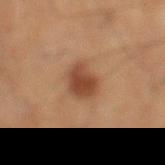Captured during whole-body skin photography for melanoma surveillance; the lesion was not biopsied. This is a cross-polarized tile. A male patient aged around 60. Automated tile analysis of the lesion measured a lesion area of about 9 mm², an outline eccentricity of about 0.7 (0 = round, 1 = elongated), and a symmetry-axis asymmetry near 0.2. The software also gave a lesion color around L≈41 a*≈20 b*≈29 in CIELAB, a lesion–skin lightness drop of about 11, and a normalized lesion–skin contrast near 8.5. And it measured a nevus-likeness score of about 95/100 and a lesion-detection confidence of about 100/100. A 15 mm close-up tile from a total-body photography series done for melanoma screening. Measured at roughly 4 mm in maximum diameter. The lesion is located on the leg.